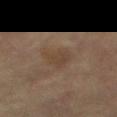The lesion's longest dimension is about 3 mm.
Captured under cross-polarized illumination.
A male subject, about 65 years old.
A close-up tile cropped from a whole-body skin photograph, about 15 mm across.
The lesion is on the abdomen.
The lesion-visualizer software estimated an area of roughly 5 mm² and an outline eccentricity of about 0.55 (0 = round, 1 = elongated). It also reported a normalized lesion–skin contrast near 5.